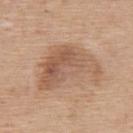follow-up: catalogued during a skin exam; not biopsied
anatomic site: the upper back
acquisition: ~15 mm tile from a whole-body skin photo
lighting: white-light illumination
patient: male, aged around 60
lesion diameter: about 7.5 mm